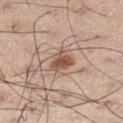Captured during whole-body skin photography for melanoma surveillance; the lesion was not biopsied.
Cropped from a whole-body photographic skin survey; the tile spans about 15 mm.
The patient is a male about 55 years old.
The lesion is located on the right thigh.
Approximately 3 mm at its widest.
Automated tile analysis of the lesion measured a shape eccentricity near 0.55 and two-axis asymmetry of about 0.35. It also reported a border-irregularity rating of about 3.5/10 and a peripheral color-asymmetry measure near 1.5. The analysis additionally found a nevus-likeness score of about 95/100 and a detector confidence of about 100 out of 100 that the crop contains a lesion.